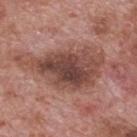Part of a total-body skin-imaging series; this lesion was reviewed on a skin check and was not flagged for biopsy. Located on the mid back. Cropped from a total-body skin-imaging series; the visible field is about 15 mm. A male patient aged around 70. The tile uses white-light illumination.